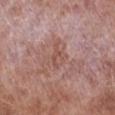No biopsy was performed on this lesion — it was imaged during a full skin examination and was not determined to be concerning. Captured under white-light illumination. On the right lower leg. A male subject, approximately 55 years of age. The lesion's longest dimension is about 3 mm. A 15 mm crop from a total-body photograph taken for skin-cancer surveillance.